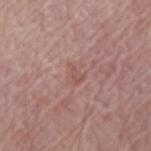Part of a total-body skin-imaging series; this lesion was reviewed on a skin check and was not flagged for biopsy.
The recorded lesion diameter is about 2.5 mm.
An algorithmic analysis of the crop reported a border-irregularity index near 4.5/10, internal color variation of about 1.5 on a 0–10 scale, and radial color variation of about 0.5. And it measured a detector confidence of about 100 out of 100 that the crop contains a lesion.
Cropped from a total-body skin-imaging series; the visible field is about 15 mm.
A female patient, in their 70s.
The lesion is on the arm.
The tile uses white-light illumination.